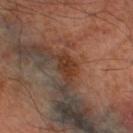biopsy status = total-body-photography surveillance lesion; no biopsy
subject = male, aged around 70
image source = ~15 mm crop, total-body skin-cancer survey
site = the right lower leg